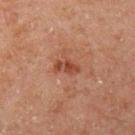Impression: Part of a total-body skin-imaging series; this lesion was reviewed on a skin check and was not flagged for biopsy. Context: The patient is a male aged approximately 45. This is a cross-polarized tile. Located on the left upper arm. The lesion-visualizer software estimated a lesion area of about 4.5 mm² and two-axis asymmetry of about 0.35. The analysis additionally found border irregularity of about 3.5 on a 0–10 scale, a within-lesion color-variation index near 3.5/10, and peripheral color asymmetry of about 1. Cropped from a total-body skin-imaging series; the visible field is about 15 mm.